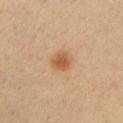| feature | finding |
|---|---|
| biopsy status | no biopsy performed (imaged during a skin exam) |
| image | ~15 mm tile from a whole-body skin photo |
| size | ~2.5 mm (longest diameter) |
| subject | male, aged 38–42 |
| site | the chest |
| automated lesion analysis | a lesion area of about 4.5 mm², a shape eccentricity near 0.45, and a symmetry-axis asymmetry near 0.25; a lesion color around L≈56 a*≈23 b*≈38 in CIELAB, about 11 CIELAB-L* units darker than the surrounding skin, and a lesion-to-skin contrast of about 8 (normalized; higher = more distinct); a peripheral color-asymmetry measure near 0.5; a classifier nevus-likeness of about 100/100 |